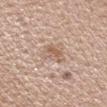No biopsy was performed on this lesion — it was imaged during a full skin examination and was not determined to be concerning. A lesion tile, about 15 mm wide, cut from a 3D total-body photograph. A male patient in their mid- to late 20s. From the head or neck.